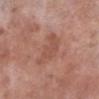biopsy_status: not biopsied; imaged during a skin examination
patient:
  sex: male
  age_approx: 75
image:
  source: total-body photography crop
  field_of_view_mm: 15
automated_metrics:
  area_mm2_approx: 9.0
  eccentricity: 0.85
  shape_asymmetry: 0.4
  color_variation_0_10: 1.5
  peripheral_color_asymmetry: 0.5
lighting: white-light
lesion_size:
  long_diameter_mm_approx: 5.0
site: leg